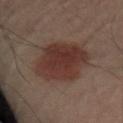The lesion was photographed on a routine skin check and not biopsied; there is no pathology result.
A close-up tile cropped from a whole-body skin photograph, about 15 mm across.
The lesion's longest dimension is about 6.5 mm.
The total-body-photography lesion software estimated a footprint of about 27 mm², an eccentricity of roughly 0.55, and two-axis asymmetry of about 0.1. And it measured an average lesion color of about L≈33 a*≈18 b*≈21 (CIELAB) and a normalized lesion–skin contrast near 9.
The patient is a male aged 63 to 67.
The lesion is located on the front of the torso.
The tile uses cross-polarized illumination.